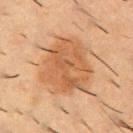No biopsy was performed on this lesion — it was imaged during a full skin examination and was not determined to be concerning.
The patient is a male aged around 55.
Measured at roughly 7 mm in maximum diameter.
A 15 mm crop from a total-body photograph taken for skin-cancer surveillance.
On the upper back.